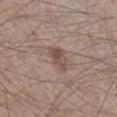Q: Was this lesion biopsied?
A: no biopsy performed (imaged during a skin exam)
Q: Lesion location?
A: the leg
Q: Who is the patient?
A: male, about 45 years old
Q: How was this image acquired?
A: 15 mm crop, total-body photography